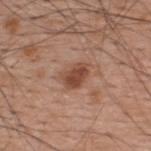This lesion was catalogued during total-body skin photography and was not selected for biopsy.
A male subject, aged approximately 60.
A 15 mm crop from a total-body photograph taken for skin-cancer surveillance.
The lesion is located on the upper back.
Automated tile analysis of the lesion measured a footprint of about 6 mm², a shape eccentricity near 0.7, and two-axis asymmetry of about 0.2. It also reported a lesion color around L≈47 a*≈23 b*≈30 in CIELAB and about 11 CIELAB-L* units darker than the surrounding skin.